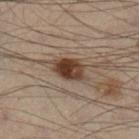Part of a total-body skin-imaging series; this lesion was reviewed on a skin check and was not flagged for biopsy.
The lesion-visualizer software estimated a mean CIELAB color near L≈31 a*≈14 b*≈21 and about 12 CIELAB-L* units darker than the surrounding skin. The software also gave internal color variation of about 5 on a 0–10 scale and a peripheral color-asymmetry measure near 2. And it measured a classifier nevus-likeness of about 100/100 and a detector confidence of about 100 out of 100 that the crop contains a lesion.
The lesion is on the right lower leg.
The lesion's longest dimension is about 4 mm.
A lesion tile, about 15 mm wide, cut from a 3D total-body photograph.
The tile uses cross-polarized illumination.
A male subject aged approximately 55.